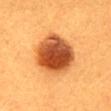Recorded during total-body skin imaging; not selected for excision or biopsy.
The recorded lesion diameter is about 6 mm.
Cropped from a whole-body photographic skin survey; the tile spans about 15 mm.
The lesion-visualizer software estimated an area of roughly 23 mm², an eccentricity of roughly 0.55, and two-axis asymmetry of about 0.1. It also reported an automated nevus-likeness rating near 100 out of 100 and a lesion-detection confidence of about 100/100.
The lesion is on the back.
This is a cross-polarized tile.
A female subject aged approximately 30.